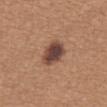Notes:
* biopsy status · catalogued during a skin exam; not biopsied
* tile lighting · white-light illumination
* anatomic site · the abdomen
* patient · female, about 45 years old
* image-analysis metrics · a footprint of about 8.5 mm² and a shape eccentricity near 0.65; a lesion color around L≈44 a*≈20 b*≈25 in CIELAB, roughly 15 lightness units darker than nearby skin, and a lesion-to-skin contrast of about 12 (normalized; higher = more distinct); internal color variation of about 6 on a 0–10 scale and peripheral color asymmetry of about 1.5; an automated nevus-likeness rating near 50 out of 100 and a detector confidence of about 100 out of 100 that the crop contains a lesion
* size · about 3.5 mm
* imaging modality · 15 mm crop, total-body photography